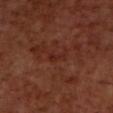biopsy status = total-body-photography surveillance lesion; no biopsy
illumination = cross-polarized illumination
site = the chest
subject = male, aged around 70
image source = ~15 mm tile from a whole-body skin photo
lesion size = about 2.5 mm